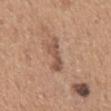<record>
  <biopsy_status>not biopsied; imaged during a skin examination</biopsy_status>
  <lesion_size>
    <long_diameter_mm_approx>4.5</long_diameter_mm_approx>
  </lesion_size>
  <image>
    <source>total-body photography crop</source>
    <field_of_view_mm>15</field_of_view_mm>
  </image>
  <site>back</site>
  <automated_metrics>
    <area_mm2_approx>5.5</area_mm2_approx>
    <shape_asymmetry>0.35</shape_asymmetry>
    <cielab_L>52</cielab_L>
    <cielab_a>20</cielab_a>
    <cielab_b>29</cielab_b>
    <vs_skin_darker_L>10.0</vs_skin_darker_L>
    <vs_skin_contrast_norm>7.5</vs_skin_contrast_norm>
    <border_irregularity_0_10>5.5</border_irregularity_0_10>
    <color_variation_0_10>3.0</color_variation_0_10>
    <peripheral_color_asymmetry>1.0</peripheral_color_asymmetry>
  </automated_metrics>
  <patient>
    <sex>male</sex>
    <age_approx>65</age_approx>
  </patient>
  <lighting>white-light</lighting>
</record>